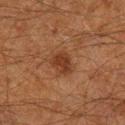workup: no biopsy performed (imaged during a skin exam) | location: the right lower leg | diameter: ~3 mm (longest diameter) | image-analysis metrics: an area of roughly 6 mm², an outline eccentricity of about 0.65 (0 = round, 1 = elongated), and a shape-asymmetry score of about 0.25 (0 = symmetric); an automated nevus-likeness rating near 55 out of 100 | subject: male, approximately 60 years of age | image: ~15 mm tile from a whole-body skin photo.